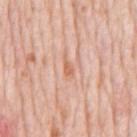The lesion was tiled from a total-body skin photograph and was not biopsied.
The lesion is located on the chest.
This is a white-light tile.
The lesion-visualizer software estimated a footprint of about 3 mm², an outline eccentricity of about 0.9 (0 = round, 1 = elongated), and a shape-asymmetry score of about 0.35 (0 = symmetric). The software also gave an average lesion color of about L≈66 a*≈23 b*≈34 (CIELAB), about 8 CIELAB-L* units darker than the surrounding skin, and a lesion-to-skin contrast of about 6.5 (normalized; higher = more distinct). It also reported a color-variation rating of about 0.5/10 and radial color variation of about 0.
A male subject, in their 80s.
Cropped from a whole-body photographic skin survey; the tile spans about 15 mm.